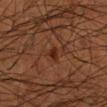Imaged during a routine full-body skin examination; the lesion was not biopsied and no histopathology is available. A roughly 15 mm field-of-view crop from a total-body skin photograph. The recorded lesion diameter is about 1.5 mm. Automated tile analysis of the lesion measured a lesion area of about 2 mm², an outline eccentricity of about 0.6 (0 = round, 1 = elongated), and a symmetry-axis asymmetry near 0.3. It also reported a lesion–skin lightness drop of about 8. A male patient aged 63–67. This is a cross-polarized tile. The lesion is on the right forearm.